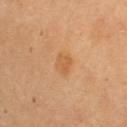Notes:
- workup — imaged on a skin check; not biopsied
- diameter — ~2.5 mm (longest diameter)
- image source — ~15 mm tile from a whole-body skin photo
- subject — female, roughly 60 years of age
- tile lighting — cross-polarized illumination
- location — the left upper arm